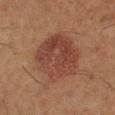{
  "biopsy_status": "not biopsied; imaged during a skin examination",
  "site": "right lower leg",
  "lesion_size": {
    "long_diameter_mm_approx": 6.0
  },
  "image": {
    "source": "total-body photography crop",
    "field_of_view_mm": 15
  },
  "patient": {
    "sex": "female",
    "age_approx": 50
  },
  "automated_metrics": {
    "area_mm2_approx": 27.0,
    "cielab_L": 34,
    "cielab_a": 19,
    "cielab_b": 23,
    "vs_skin_darker_L": 7.0,
    "vs_skin_contrast_norm": 7.0,
    "border_irregularity_0_10": 1.5,
    "color_variation_0_10": 4.0
  }
}